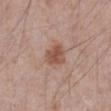Imaged during a routine full-body skin examination; the lesion was not biopsied and no histopathology is available.
Cropped from a whole-body photographic skin survey; the tile spans about 15 mm.
The recorded lesion diameter is about 3 mm.
The lesion is located on the abdomen.
Captured under white-light illumination.
A male patient approximately 70 years of age.